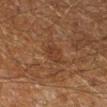This lesion was catalogued during total-body skin photography and was not selected for biopsy.
The lesion-visualizer software estimated a footprint of about 4 mm² and a shape-asymmetry score of about 0.3 (0 = symmetric). The analysis additionally found a lesion color around L≈28 a*≈17 b*≈24 in CIELAB and a lesion–skin lightness drop of about 5. It also reported a within-lesion color-variation index near 1.5/10 and radial color variation of about 0.5. The analysis additionally found a detector confidence of about 100 out of 100 that the crop contains a lesion.
Located on the right lower leg.
This is a cross-polarized tile.
The lesion's longest dimension is about 2.5 mm.
The subject is a male aged around 60.
A 15 mm crop from a total-body photograph taken for skin-cancer surveillance.